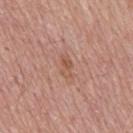Q: Was this lesion biopsied?
A: catalogued during a skin exam; not biopsied
Q: What did automated image analysis measure?
A: an average lesion color of about L≈54 a*≈22 b*≈30 (CIELAB) and roughly 7 lightness units darker than nearby skin; a border-irregularity index near 4/10, a color-variation rating of about 0.5/10, and a peripheral color-asymmetry measure near 0; a nevus-likeness score of about 0/100 and lesion-presence confidence of about 100/100
Q: How was the tile lit?
A: white-light
Q: What is the anatomic site?
A: the mid back
Q: Who is the patient?
A: male, aged 58–62
Q: How was this image acquired?
A: total-body-photography crop, ~15 mm field of view
Q: What is the lesion's diameter?
A: about 2.5 mm